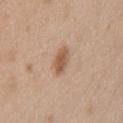biopsy status: imaged on a skin check; not biopsied
automated lesion analysis: an average lesion color of about L≈56 a*≈19 b*≈32 (CIELAB) and roughly 11 lightness units darker than nearby skin; an automated nevus-likeness rating near 90 out of 100 and a detector confidence of about 100 out of 100 that the crop contains a lesion
anatomic site: the chest
patient: female, aged 38–42
tile lighting: white-light
acquisition: 15 mm crop, total-body photography